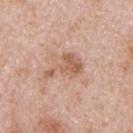Part of a total-body skin-imaging series; this lesion was reviewed on a skin check and was not flagged for biopsy. The lesion-visualizer software estimated an area of roughly 8 mm² and a shape eccentricity near 0.8. It also reported a nevus-likeness score of about 0/100. Approximately 4.5 mm at its widest. On the back. Captured under white-light illumination. A close-up tile cropped from a whole-body skin photograph, about 15 mm across. A female patient aged 43–47.